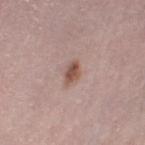The lesion was tiled from a total-body skin photograph and was not biopsied. Cropped from a whole-body photographic skin survey; the tile spans about 15 mm. A female patient in their 50s. From the left lower leg.